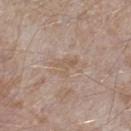Q: Was a biopsy performed?
A: catalogued during a skin exam; not biopsied
Q: Lesion location?
A: the leg
Q: What lighting was used for the tile?
A: white-light
Q: What did automated image analysis measure?
A: a footprint of about 4.5 mm², an eccentricity of roughly 0.75, and a shape-asymmetry score of about 0.55 (0 = symmetric); an average lesion color of about L≈57 a*≈15 b*≈27 (CIELAB), about 6 CIELAB-L* units darker than the surrounding skin, and a normalized lesion–skin contrast near 5
Q: Who is the patient?
A: male, in their mid- to late 40s
Q: How was this image acquired?
A: 15 mm crop, total-body photography